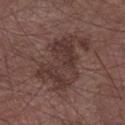Assessment: Imaged during a routine full-body skin examination; the lesion was not biopsied and no histopathology is available. Acquisition and patient details: A male subject, aged 73 to 77. Automated image analysis of the tile measured border irregularity of about 7 on a 0–10 scale, internal color variation of about 4 on a 0–10 scale, and a peripheral color-asymmetry measure near 1.5. The lesion is located on the arm. A 15 mm crop from a total-body photograph taken for skin-cancer surveillance.